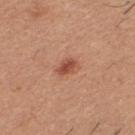workup: no biopsy performed (imaged during a skin exam) | subject: male, in their 60s | TBP lesion metrics: an area of roughly 4 mm², a shape eccentricity near 0.8, and a shape-asymmetry score of about 0.2 (0 = symmetric); border irregularity of about 2 on a 0–10 scale, a within-lesion color-variation index near 2.5/10, and peripheral color asymmetry of about 1; an automated nevus-likeness rating near 90 out of 100 and a detector confidence of about 100 out of 100 that the crop contains a lesion | lighting: white-light | lesion diameter: ≈3 mm | image: ~15 mm crop, total-body skin-cancer survey | site: the upper back.